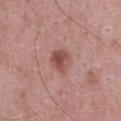Part of a total-body skin-imaging series; this lesion was reviewed on a skin check and was not flagged for biopsy. The lesion-visualizer software estimated an outline eccentricity of about 0.25 (0 = round, 1 = elongated) and a symmetry-axis asymmetry near 0.25. And it measured a nevus-likeness score of about 75/100 and lesion-presence confidence of about 100/100. Measured at roughly 3 mm in maximum diameter. A male subject, aged approximately 25. Cropped from a total-body skin-imaging series; the visible field is about 15 mm. The lesion is located on the upper back.